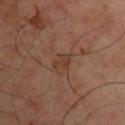- follow-up: total-body-photography surveillance lesion; no biopsy
- image source: ~15 mm crop, total-body skin-cancer survey
- location: the chest
- subject: male, aged 48–52